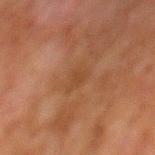Q: Was this lesion biopsied?
A: catalogued during a skin exam; not biopsied